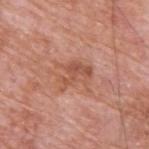Assessment: No biopsy was performed on this lesion — it was imaged during a full skin examination and was not determined to be concerning. Acquisition and patient details: This is a white-light tile. Automated image analysis of the tile measured an area of roughly 7.5 mm², an eccentricity of roughly 0.7, and a shape-asymmetry score of about 0.45 (0 = symmetric). And it measured an average lesion color of about L≈54 a*≈25 b*≈31 (CIELAB), a lesion–skin lightness drop of about 8, and a lesion-to-skin contrast of about 6 (normalized; higher = more distinct). Located on the upper back. A 15 mm crop from a total-body photograph taken for skin-cancer surveillance. A male patient in their 60s.